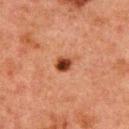Assessment: Captured during whole-body skin photography for melanoma surveillance; the lesion was not biopsied. Acquisition and patient details: A male patient, aged around 50. The recorded lesion diameter is about 2 mm. Imaged with cross-polarized lighting. The lesion is on the upper back. This image is a 15 mm lesion crop taken from a total-body photograph. The total-body-photography lesion software estimated internal color variation of about 5 on a 0–10 scale and a peripheral color-asymmetry measure near 2.